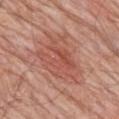This lesion was catalogued during total-body skin photography and was not selected for biopsy.
The total-body-photography lesion software estimated a footprint of about 21 mm² and a symmetry-axis asymmetry near 0.35. The analysis additionally found a lesion color around L≈53 a*≈27 b*≈29 in CIELAB, a lesion–skin lightness drop of about 8, and a normalized border contrast of about 5.5.
A male subject, about 80 years old.
This is a white-light tile.
On the mid back.
Cropped from a whole-body photographic skin survey; the tile spans about 15 mm.